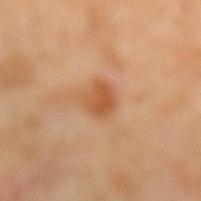No biopsy was performed on this lesion — it was imaged during a full skin examination and was not determined to be concerning. A roughly 15 mm field-of-view crop from a total-body skin photograph. The lesion is on the left lower leg. A female subject about 50 years old.